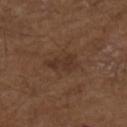biopsy status — imaged on a skin check; not biopsied
subject — male, in their mid- to late 70s
body site — the arm
imaging modality — total-body-photography crop, ~15 mm field of view